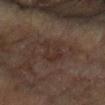Notes:
* follow-up · total-body-photography surveillance lesion; no biopsy
* anatomic site · the arm
* TBP lesion metrics · internal color variation of about 2 on a 0–10 scale and peripheral color asymmetry of about 0.5
* patient · female, roughly 80 years of age
* acquisition · ~15 mm tile from a whole-body skin photo